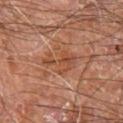Notes:
– notes · no biopsy performed (imaged during a skin exam)
– body site · the leg
– subject · male, about 60 years old
– image · ~15 mm tile from a whole-body skin photo
– lesion diameter · ≈4 mm
– lighting · cross-polarized illumination